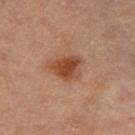biopsy status = catalogued during a skin exam; not biopsied | imaging modality = total-body-photography crop, ~15 mm field of view | anatomic site = the left thigh | image-analysis metrics = a nevus-likeness score of about 95/100 and a detector confidence of about 100 out of 100 that the crop contains a lesion | tile lighting = cross-polarized illumination | lesion size = ~4 mm (longest diameter) | patient = male, approximately 85 years of age or older.